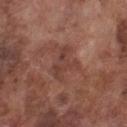notes: no biopsy performed (imaged during a skin exam) | illumination: white-light illumination | image source: ~15 mm tile from a whole-body skin photo | size: about 4.5 mm | automated lesion analysis: a shape eccentricity near 0.75 and a symmetry-axis asymmetry near 0.5; a lesion color around L≈41 a*≈22 b*≈25 in CIELAB, roughly 7 lightness units darker than nearby skin, and a lesion-to-skin contrast of about 6 (normalized; higher = more distinct); a classifier nevus-likeness of about 0/100 and a lesion-detection confidence of about 100/100 | location: the chest | patient: male, approximately 75 years of age.